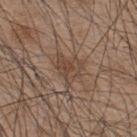The lesion was photographed on a routine skin check and not biopsied; there is no pathology result.
The total-body-photography lesion software estimated a lesion area of about 7.5 mm², an outline eccentricity of about 0.5 (0 = round, 1 = elongated), and two-axis asymmetry of about 0.5. The software also gave an average lesion color of about L≈44 a*≈16 b*≈26 (CIELAB), a lesion–skin lightness drop of about 7, and a normalized border contrast of about 6. It also reported internal color variation of about 3.5 on a 0–10 scale and peripheral color asymmetry of about 1. The software also gave a nevus-likeness score of about 5/100 and lesion-presence confidence of about 95/100.
The recorded lesion diameter is about 4 mm.
A male patient, in their mid-40s.
A 15 mm close-up extracted from a 3D total-body photography capture.
The lesion is on the back.
This is a white-light tile.